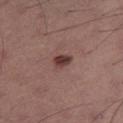{"biopsy_status": "not biopsied; imaged during a skin examination", "image": {"source": "total-body photography crop", "field_of_view_mm": 15}, "patient": {"sex": "male", "age_approx": 40}, "site": "right thigh", "automated_metrics": {"shape_asymmetry": 0.3}}